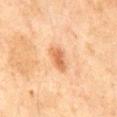Imaged during a routine full-body skin examination; the lesion was not biopsied and no histopathology is available.
Approximately 3 mm at its widest.
A male patient, approximately 45 years of age.
On the mid back.
A region of skin cropped from a whole-body photographic capture, roughly 15 mm wide.